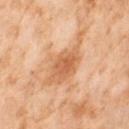No biopsy was performed on this lesion — it was imaged during a full skin examination and was not determined to be concerning.
The lesion is located on the right thigh.
Longest diameter approximately 7 mm.
An algorithmic analysis of the crop reported a footprint of about 14 mm², a shape eccentricity near 0.85, and a shape-asymmetry score of about 0.4 (0 = symmetric). And it measured a border-irregularity index near 6.5/10 and internal color variation of about 4 on a 0–10 scale.
A female patient roughly 55 years of age.
A 15 mm close-up extracted from a 3D total-body photography capture.